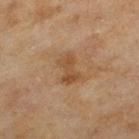Captured during whole-body skin photography for melanoma surveillance; the lesion was not biopsied. On the left thigh. A female patient, aged 58 to 62. A region of skin cropped from a whole-body photographic capture, roughly 15 mm wide.